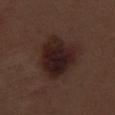No biopsy was performed on this lesion — it was imaged during a full skin examination and was not determined to be concerning. A male subject, about 70 years old. Imaged with white-light lighting. Automated tile analysis of the lesion measured an area of roughly 21 mm² and two-axis asymmetry of about 0.25. And it measured a classifier nevus-likeness of about 85/100 and lesion-presence confidence of about 100/100. From the right thigh. About 6 mm across. A roughly 15 mm field-of-view crop from a total-body skin photograph.